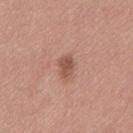Q: Was this lesion biopsied?
A: catalogued during a skin exam; not biopsied
Q: Patient demographics?
A: female, about 40 years old
Q: What is the imaging modality?
A: 15 mm crop, total-body photography
Q: Where on the body is the lesion?
A: the left thigh
Q: Illumination type?
A: white-light illumination